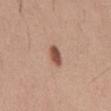Assessment:
Part of a total-body skin-imaging series; this lesion was reviewed on a skin check and was not flagged for biopsy.
Clinical summary:
Captured under white-light illumination. The lesion's longest dimension is about 2.5 mm. From the abdomen. A close-up tile cropped from a whole-body skin photograph, about 15 mm across. A male subject aged around 30.